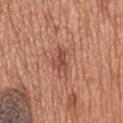workup — imaged on a skin check; not biopsied
image — ~15 mm crop, total-body skin-cancer survey
subject — male, in their mid-60s
body site — the mid back